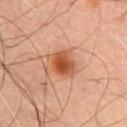<lesion>
<lesion_size>
  <long_diameter_mm_approx>3.5</long_diameter_mm_approx>
</lesion_size>
<patient>
  <sex>male</sex>
  <age_approx>55</age_approx>
</patient>
<site>chest</site>
<lighting>cross-polarized</lighting>
<image>
  <source>total-body photography crop</source>
  <field_of_view_mm>15</field_of_view_mm>
</image>
</lesion>